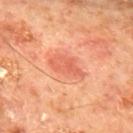Imaged during a routine full-body skin examination; the lesion was not biopsied and no histopathology is available. The patient is a male in their mid- to late 60s. A region of skin cropped from a whole-body photographic capture, roughly 15 mm wide. Located on the mid back.